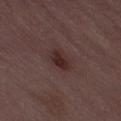The recorded lesion diameter is about 3.5 mm. The lesion-visualizer software estimated an automated nevus-likeness rating near 80 out of 100. A male patient, aged around 50. Captured under white-light illumination. A 15 mm close-up tile from a total-body photography series done for melanoma screening.